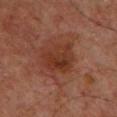The lesion was photographed on a routine skin check and not biopsied; there is no pathology result. A male subject, approximately 60 years of age. On the upper back. A roughly 15 mm field-of-view crop from a total-body skin photograph.